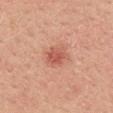Acquisition and patient details:
The patient is a female aged 43 to 47. On the upper back. A roughly 15 mm field-of-view crop from a total-body skin photograph. Captured under white-light illumination. Approximately 3.5 mm at its widest.